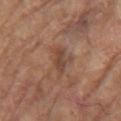Assessment: The lesion was photographed on a routine skin check and not biopsied; there is no pathology result. Image and clinical context: Cropped from a total-body skin-imaging series; the visible field is about 15 mm. The patient is a male aged around 80. On the left upper arm. This is a white-light tile. Automated image analysis of the tile measured a lesion area of about 4.5 mm², a shape eccentricity near 0.85, and a shape-asymmetry score of about 0.3 (0 = symmetric). The analysis additionally found a lesion color around L≈44 a*≈20 b*≈27 in CIELAB, about 8 CIELAB-L* units darker than the surrounding skin, and a normalized border contrast of about 6.5. It also reported a within-lesion color-variation index near 2/10 and a peripheral color-asymmetry measure near 0.5.